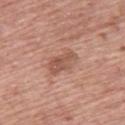biopsy status: imaged on a skin check; not biopsied | tile lighting: white-light illumination | acquisition: ~15 mm crop, total-body skin-cancer survey | subject: male, aged 58–62 | anatomic site: the back | lesion diameter: ~4 mm (longest diameter) | image-analysis metrics: a mean CIELAB color near L≈54 a*≈22 b*≈29, about 9 CIELAB-L* units darker than the surrounding skin, and a normalized lesion–skin contrast near 6.5.